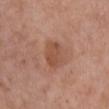follow-up: imaged on a skin check; not biopsied
acquisition: 15 mm crop, total-body photography
patient: female, in their mid- to late 60s
location: the chest